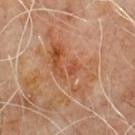notes: imaged on a skin check; not biopsied | illumination: cross-polarized illumination | TBP lesion metrics: an eccentricity of roughly 0.9 and a shape-asymmetry score of about 0.4 (0 = symmetric); a lesion color around L≈51 a*≈23 b*≈34 in CIELAB and about 7 CIELAB-L* units darker than the surrounding skin; a within-lesion color-variation index near 9/10 and peripheral color asymmetry of about 3; lesion-presence confidence of about 100/100 | body site: the upper back | acquisition: ~15 mm tile from a whole-body skin photo | subject: male, in their 60s | lesion diameter: about 8.5 mm.